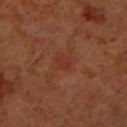This image is a 15 mm lesion crop taken from a total-body photograph. Longest diameter approximately 2.5 mm. From the left forearm. Captured under cross-polarized illumination. Automated tile analysis of the lesion measured a lesion–skin lightness drop of about 5. And it measured border irregularity of about 2 on a 0–10 scale, a color-variation rating of about 0.5/10, and a peripheral color-asymmetry measure near 0. The analysis additionally found a classifier nevus-likeness of about 0/100. The patient is a female aged approximately 50.